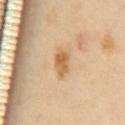The lesion was photographed on a routine skin check and not biopsied; there is no pathology result. From the chest. The subject is a female roughly 55 years of age. This is a cross-polarized tile. Measured at roughly 2.5 mm in maximum diameter. A 15 mm close-up extracted from a 3D total-body photography capture. An algorithmic analysis of the crop reported a lesion area of about 4.5 mm² and an outline eccentricity of about 0.7 (0 = round, 1 = elongated).